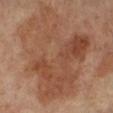follow-up = catalogued during a skin exam; not biopsied
imaging modality = ~15 mm tile from a whole-body skin photo
patient = female, in their mid-60s
diameter = about 11.5 mm
body site = the left leg